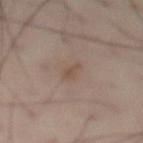Assessment:
Imaged during a routine full-body skin examination; the lesion was not biopsied and no histopathology is available.